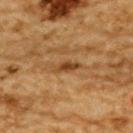Assessment:
Part of a total-body skin-imaging series; this lesion was reviewed on a skin check and was not flagged for biopsy.
Clinical summary:
The lesion-visualizer software estimated an average lesion color of about L≈38 a*≈19 b*≈34 (CIELAB), a lesion–skin lightness drop of about 9, and a normalized lesion–skin contrast near 8. And it measured a border-irregularity rating of about 3.5/10 and a within-lesion color-variation index near 1/10. The software also gave an automated nevus-likeness rating near 20 out of 100 and a detector confidence of about 95 out of 100 that the crop contains a lesion. This image is a 15 mm lesion crop taken from a total-body photograph. Measured at roughly 3 mm in maximum diameter. A male patient aged 83 or older. Located on the upper back.